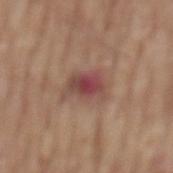Assessment: The lesion was photographed on a routine skin check and not biopsied; there is no pathology result. Clinical summary: Imaged with white-light lighting. A male patient, about 75 years old. Approximately 4 mm at its widest. Cropped from a whole-body photographic skin survey; the tile spans about 15 mm. Automated tile analysis of the lesion measured a symmetry-axis asymmetry near 0.3. It also reported an average lesion color of about L≈47 a*≈22 b*≈23 (CIELAB) and a lesion–skin lightness drop of about 10. The analysis additionally found a nevus-likeness score of about 0/100 and a lesion-detection confidence of about 100/100. The lesion is located on the mid back.